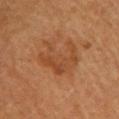Clinical impression: The lesion was tiled from a total-body skin photograph and was not biopsied. Image and clinical context: From the upper back. A lesion tile, about 15 mm wide, cut from a 3D total-body photograph. The tile uses cross-polarized illumination. A female patient, aged around 65.